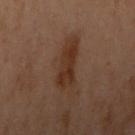This lesion was catalogued during total-body skin photography and was not selected for biopsy.
A roughly 15 mm field-of-view crop from a total-body skin photograph.
Automated tile analysis of the lesion measured a lesion area of about 9 mm² and a shape eccentricity near 0.9. The analysis additionally found a lesion–skin lightness drop of about 7 and a normalized border contrast of about 8. The software also gave a border-irregularity rating of about 3.5/10 and a within-lesion color-variation index near 3/10.
Located on the left arm.
A female subject approximately 55 years of age.